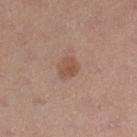Context:
A roughly 15 mm field-of-view crop from a total-body skin photograph. The patient is a female in their 30s. Imaged with white-light lighting. On the leg.